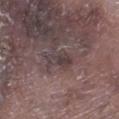location = the leg; lighting = white-light; subject = male, aged around 75; image = 15 mm crop, total-body photography.